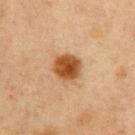biopsy status — catalogued during a skin exam; not biopsied | patient — male, approximately 75 years of age | anatomic site — the chest | acquisition — total-body-photography crop, ~15 mm field of view | lesion size — ≈3.5 mm | tile lighting — cross-polarized | automated metrics — border irregularity of about 1.5 on a 0–10 scale, a within-lesion color-variation index near 4/10, and radial color variation of about 1.